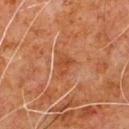No biopsy was performed on this lesion — it was imaged during a full skin examination and was not determined to be concerning. The subject is a male aged 78 to 82. The lesion is located on the front of the torso. A region of skin cropped from a whole-body photographic capture, roughly 15 mm wide. Measured at roughly 3 mm in maximum diameter. The total-body-photography lesion software estimated an area of roughly 4.5 mm², an eccentricity of roughly 0.7, and two-axis asymmetry of about 0.35. The software also gave a lesion color around L≈37 a*≈23 b*≈31 in CIELAB, roughly 6 lightness units darker than nearby skin, and a lesion-to-skin contrast of about 6 (normalized; higher = more distinct). Imaged with cross-polarized lighting.